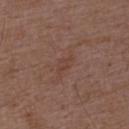Q: Is there a histopathology result?
A: imaged on a skin check; not biopsied
Q: Lesion location?
A: the upper back
Q: Patient demographics?
A: male, in their 50s
Q: What is the imaging modality?
A: 15 mm crop, total-body photography